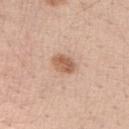Recorded during total-body skin imaging; not selected for excision or biopsy. The total-body-photography lesion software estimated a shape eccentricity near 0.7 and a symmetry-axis asymmetry near 0.2. The software also gave a lesion color around L≈59 a*≈21 b*≈32 in CIELAB and a lesion–skin lightness drop of about 12. It also reported a classifier nevus-likeness of about 95/100 and a lesion-detection confidence of about 100/100. The lesion is on the arm. The recorded lesion diameter is about 3 mm. A female patient, about 40 years old. A region of skin cropped from a whole-body photographic capture, roughly 15 mm wide.